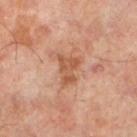notes: imaged on a skin check; not biopsied
body site: the left lower leg
image source: total-body-photography crop, ~15 mm field of view
subject: male, aged approximately 50
size: ≈3.5 mm
image-analysis metrics: about 10 CIELAB-L* units darker than the surrounding skin